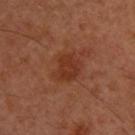This lesion was catalogued during total-body skin photography and was not selected for biopsy.
Cropped from a whole-body photographic skin survey; the tile spans about 15 mm.
The subject is a male about 30 years old.
From the upper back.